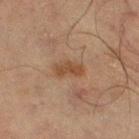Notes:
* biopsy status: no biopsy performed (imaged during a skin exam)
* site: the left thigh
* subject: male, in their mid-60s
* TBP lesion metrics: a lesion area of about 4 mm², a shape eccentricity near 0.85, and a shape-asymmetry score of about 0.35 (0 = symmetric); an average lesion color of about L≈37 a*≈17 b*≈27 (CIELAB), a lesion–skin lightness drop of about 7, and a normalized lesion–skin contrast near 7.5; a border-irregularity index near 3.5/10, a within-lesion color-variation index near 1.5/10, and a peripheral color-asymmetry measure near 0.5
* image source: ~15 mm crop, total-body skin-cancer survey